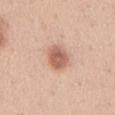The lesion was photographed on a routine skin check and not biopsied; there is no pathology result.
A male subject approximately 30 years of age.
From the chest.
A 15 mm close-up extracted from a 3D total-body photography capture.
The tile uses white-light illumination.
The lesion-visualizer software estimated a lesion color around L≈61 a*≈23 b*≈29 in CIELAB and a lesion–skin lightness drop of about 14. It also reported border irregularity of about 1.5 on a 0–10 scale, a within-lesion color-variation index near 3/10, and radial color variation of about 1. And it measured a nevus-likeness score of about 95/100.